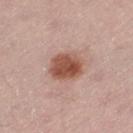Acquisition and patient details: Captured under white-light illumination. The lesion is located on the left thigh. A 15 mm close-up extracted from a 3D total-body photography capture. Longest diameter approximately 4 mm. A male subject, roughly 65 years of age.